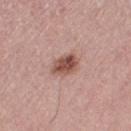Captured during whole-body skin photography for melanoma surveillance; the lesion was not biopsied.
The tile uses white-light illumination.
The lesion's longest dimension is about 3.5 mm.
The subject is a female roughly 70 years of age.
The lesion is on the leg.
A lesion tile, about 15 mm wide, cut from a 3D total-body photograph.